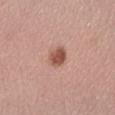{"biopsy_status": "not biopsied; imaged during a skin examination", "site": "right forearm", "image": {"source": "total-body photography crop", "field_of_view_mm": 15}, "lesion_size": {"long_diameter_mm_approx": 3.0}, "patient": {"sex": "female", "age_approx": 50}, "lighting": "white-light"}